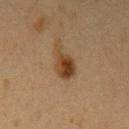notes=imaged on a skin check; not biopsied
site=the left upper arm
acquisition=15 mm crop, total-body photography
patient=female, aged approximately 40